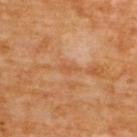Findings:
• notes: no biopsy performed (imaged during a skin exam)
• diameter: about 2.5 mm
• automated metrics: an eccentricity of roughly 0.9 and a symmetry-axis asymmetry near 0.4; border irregularity of about 4 on a 0–10 scale, a within-lesion color-variation index near 0/10, and peripheral color asymmetry of about 0
• imaging modality: total-body-photography crop, ~15 mm field of view
• patient: male, aged 58 to 62
• location: the upper back
• tile lighting: cross-polarized illumination